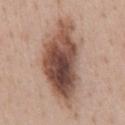workup=imaged on a skin check; not biopsied | diameter=about 10.5 mm | lighting=white-light | body site=the abdomen | patient=female, about 45 years old | TBP lesion metrics=a lesion area of about 37 mm², a shape eccentricity near 0.9, and two-axis asymmetry of about 0.25; a lesion color around L≈50 a*≈19 b*≈26 in CIELAB, roughly 17 lightness units darker than nearby skin, and a normalized border contrast of about 11.5; a border-irregularity index near 3.5/10 and a color-variation rating of about 10/10 | acquisition=~15 mm crop, total-body skin-cancer survey.